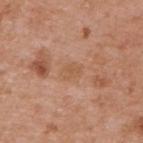Q: Was a biopsy performed?
A: no biopsy performed (imaged during a skin exam)
Q: What are the patient's age and sex?
A: male, aged approximately 55
Q: How was this image acquired?
A: ~15 mm crop, total-body skin-cancer survey
Q: Automated lesion metrics?
A: an area of roughly 3.5 mm², an outline eccentricity of about 0.7 (0 = round, 1 = elongated), and two-axis asymmetry of about 0.3; a mean CIELAB color near L≈56 a*≈21 b*≈33 and a normalized border contrast of about 5; a peripheral color-asymmetry measure near 0.5
Q: Lesion location?
A: the upper back
Q: Illumination type?
A: white-light
Q: Lesion size?
A: ~2.5 mm (longest diameter)